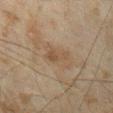<record>
<image>
  <source>total-body photography crop</source>
  <field_of_view_mm>15</field_of_view_mm>
</image>
<site>right forearm</site>
<lesion_size>
  <long_diameter_mm_approx>4.0</long_diameter_mm_approx>
</lesion_size>
<patient>
  <sex>male</sex>
  <age_approx>45</age_approx>
</patient>
</record>